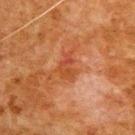Image and clinical context:
A close-up tile cropped from a whole-body skin photograph, about 15 mm across. A male patient in their 80s. Captured under cross-polarized illumination. On the upper back.